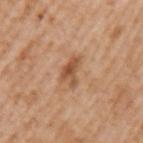notes — imaged on a skin check; not biopsied